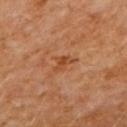{
  "biopsy_status": "not biopsied; imaged during a skin examination"
}